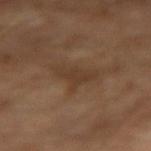  biopsy_status: not biopsied; imaged during a skin examination
  patient:
    sex: male
    age_approx: 65
  site: right forearm
  image:
    source: total-body photography crop
    field_of_view_mm: 15